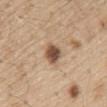Imaged during a routine full-body skin examination; the lesion was not biopsied and no histopathology is available. A male subject, roughly 70 years of age. This is a white-light tile. A 15 mm crop from a total-body photograph taken for skin-cancer surveillance. From the abdomen.